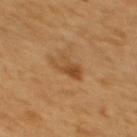Image and clinical context: About 3.5 mm across. Automated image analysis of the tile measured a shape-asymmetry score of about 0.4 (0 = symmetric). The software also gave a border-irregularity rating of about 5/10, a within-lesion color-variation index near 4/10, and peripheral color asymmetry of about 1. And it measured a detector confidence of about 100 out of 100 that the crop contains a lesion. The subject is a female approximately 55 years of age. The lesion is on the upper back. Cropped from a whole-body photographic skin survey; the tile spans about 15 mm. Imaged with cross-polarized lighting.